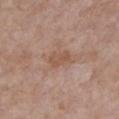Captured during whole-body skin photography for melanoma surveillance; the lesion was not biopsied. The lesion's longest dimension is about 3 mm. The lesion is located on the chest. A female subject, about 85 years old or older. A region of skin cropped from a whole-body photographic capture, roughly 15 mm wide.